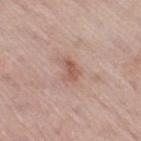Clinical impression:
Imaged during a routine full-body skin examination; the lesion was not biopsied and no histopathology is available.
Background:
Approximately 2.5 mm at its widest. Located on the leg. This image is a 15 mm lesion crop taken from a total-body photograph. Captured under white-light illumination. A male patient, approximately 60 years of age.